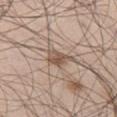Impression: Part of a total-body skin-imaging series; this lesion was reviewed on a skin check and was not flagged for biopsy. Clinical summary: On the left thigh. A male patient aged approximately 45. This image is a 15 mm lesion crop taken from a total-body photograph.